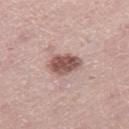Assessment:
This lesion was catalogued during total-body skin photography and was not selected for biopsy.
Image and clinical context:
From the right thigh. Cropped from a whole-body photographic skin survey; the tile spans about 15 mm. The patient is a female approximately 40 years of age.